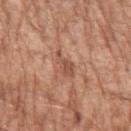<record>
  <biopsy_status>not biopsied; imaged during a skin examination</biopsy_status>
  <automated_metrics>
    <cielab_L>52</cielab_L>
    <cielab_a>23</cielab_a>
    <cielab_b>30</cielab_b>
    <vs_skin_darker_L>9.0</vs_skin_darker_L>
    <vs_skin_contrast_norm>6.5</vs_skin_contrast_norm>
    <nevus_likeness_0_100>5</nevus_likeness_0_100>
  </automated_metrics>
  <patient>
    <sex>male</sex>
    <age_approx>65</age_approx>
  </patient>
  <image>
    <source>total-body photography crop</source>
    <field_of_view_mm>15</field_of_view_mm>
  </image>
  <site>left upper arm</site>
  <lighting>white-light</lighting>
  <lesion_size>
    <long_diameter_mm_approx>3.5</long_diameter_mm_approx>
  </lesion_size>
</record>